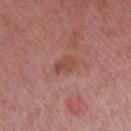Recorded during total-body skin imaging; not selected for excision or biopsy. Cropped from a total-body skin-imaging series; the visible field is about 15 mm. Located on the left upper arm. The tile uses white-light illumination. A male subject aged approximately 55.